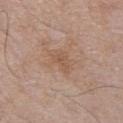The lesion was photographed on a routine skin check and not biopsied; there is no pathology result.
A male subject, aged around 55.
The total-body-photography lesion software estimated a lesion color around L≈55 a*≈18 b*≈29 in CIELAB, roughly 6 lightness units darker than nearby skin, and a normalized border contrast of about 5.
The lesion's longest dimension is about 3.5 mm.
A lesion tile, about 15 mm wide, cut from a 3D total-body photograph.
On the chest.
Captured under white-light illumination.